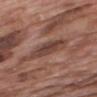Notes:
– tile lighting: white-light
– subject: male, roughly 70 years of age
– imaging modality: ~15 mm tile from a whole-body skin photo
– anatomic site: the upper back
– lesion size: about 4.5 mm